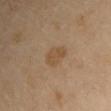This lesion was catalogued during total-body skin photography and was not selected for biopsy.
A male patient about 70 years old.
The total-body-photography lesion software estimated a border-irregularity rating of about 3/10, a color-variation rating of about 1.5/10, and peripheral color asymmetry of about 0.5.
The lesion is located on the left upper arm.
Measured at roughly 3 mm in maximum diameter.
A lesion tile, about 15 mm wide, cut from a 3D total-body photograph.